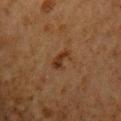Context: A lesion tile, about 15 mm wide, cut from a 3D total-body photograph. The patient is a male aged around 60. Automated image analysis of the tile measured a lesion area of about 3.5 mm², a shape eccentricity near 0.9, and two-axis asymmetry of about 0.4. The software also gave an automated nevus-likeness rating near 45 out of 100. On the chest. Imaged with cross-polarized lighting. Longest diameter approximately 3 mm.